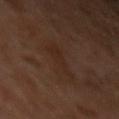{"biopsy_status": "not biopsied; imaged during a skin examination", "patient": {"sex": "male", "age_approx": 30}, "site": "left forearm", "image": {"source": "total-body photography crop", "field_of_view_mm": 15}}